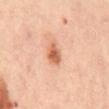Q: Is there a histopathology result?
A: no biopsy performed (imaged during a skin exam)